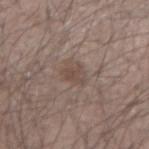Q: Was this lesion biopsied?
A: total-body-photography surveillance lesion; no biopsy
Q: What are the patient's age and sex?
A: male, aged approximately 45
Q: What is the imaging modality?
A: ~15 mm tile from a whole-body skin photo
Q: Illumination type?
A: white-light illumination
Q: Automated lesion metrics?
A: a footprint of about 4 mm², an eccentricity of roughly 0.75, and two-axis asymmetry of about 0.35
Q: Lesion location?
A: the left forearm